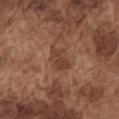Captured during whole-body skin photography for melanoma surveillance; the lesion was not biopsied.
A close-up tile cropped from a whole-body skin photograph, about 15 mm across.
This is a white-light tile.
The lesion is on the left upper arm.
A male patient aged 73 to 77.